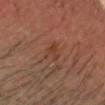biopsy status = no biopsy performed (imaged during a skin exam)
body site = the head or neck
subject = male, aged 33–37
acquisition = total-body-photography crop, ~15 mm field of view